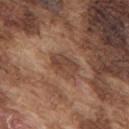{"biopsy_status": "not biopsied; imaged during a skin examination", "site": "upper back", "image": {"source": "total-body photography crop", "field_of_view_mm": 15}, "lesion_size": {"long_diameter_mm_approx": 3.5}, "patient": {"sex": "male", "age_approx": 75}, "automated_metrics": {"vs_skin_darker_L": 8.0, "vs_skin_contrast_norm": 6.5, "color_variation_0_10": 3.5, "peripheral_color_asymmetry": 1.0}, "lighting": "white-light"}